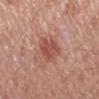workup: total-body-photography surveillance lesion; no biopsy
subject: female, roughly 50 years of age
body site: the left forearm
automated metrics: an average lesion color of about L≈51 a*≈26 b*≈27 (CIELAB); internal color variation of about 3 on a 0–10 scale and a peripheral color-asymmetry measure near 1; an automated nevus-likeness rating near 65 out of 100 and a lesion-detection confidence of about 100/100
image: ~15 mm crop, total-body skin-cancer survey